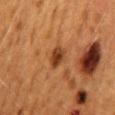Part of a total-body skin-imaging series; this lesion was reviewed on a skin check and was not flagged for biopsy. The lesion's longest dimension is about 3 mm. This is a cross-polarized tile. A female patient aged approximately 50. Cropped from a total-body skin-imaging series; the visible field is about 15 mm. Located on the back.